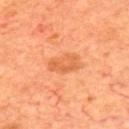<case>
  <biopsy_status>not biopsied; imaged during a skin examination</biopsy_status>
  <patient>
    <sex>male</sex>
    <age_approx>70</age_approx>
  </patient>
  <site>upper back</site>
  <image>
    <source>total-body photography crop</source>
    <field_of_view_mm>15</field_of_view_mm>
  </image>
</case>